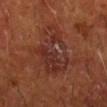{"biopsy_status": "not biopsied; imaged during a skin examination", "lesion_size": {"long_diameter_mm_approx": 7.0}, "patient": {"sex": "male", "age_approx": 60}, "lighting": "cross-polarized", "image": {"source": "total-body photography crop", "field_of_view_mm": 15}, "automated_metrics": {"shape_asymmetry": 0.35, "border_irregularity_0_10": 6.5, "peripheral_color_asymmetry": 2.0}, "site": "right forearm"}